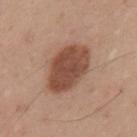Impression: The lesion was photographed on a routine skin check and not biopsied; there is no pathology result. Clinical summary: The lesion is located on the mid back. Captured under white-light illumination. A male subject, in their mid- to late 30s. The recorded lesion diameter is about 6 mm. A roughly 15 mm field-of-view crop from a total-body skin photograph. Automated image analysis of the tile measured an average lesion color of about L≈48 a*≈22 b*≈28 (CIELAB), a lesion–skin lightness drop of about 14, and a normalized lesion–skin contrast near 9.5. And it measured a border-irregularity rating of about 1.5/10, a color-variation rating of about 3.5/10, and a peripheral color-asymmetry measure near 1.